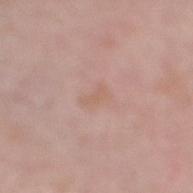Captured during whole-body skin photography for melanoma surveillance; the lesion was not biopsied. A 15 mm crop from a total-body photograph taken for skin-cancer surveillance. Automated image analysis of the tile measured a lesion area of about 3 mm², an outline eccentricity of about 0.9 (0 = round, 1 = elongated), and a symmetry-axis asymmetry near 0.35. It also reported an average lesion color of about L≈61 a*≈19 b*≈27 (CIELAB), a lesion–skin lightness drop of about 5, and a normalized border contrast of about 4.5. The analysis additionally found border irregularity of about 4 on a 0–10 scale, internal color variation of about 0 on a 0–10 scale, and radial color variation of about 0. From the right lower leg. The patient is a male aged 68 to 72. This is a white-light tile. About 3 mm across.